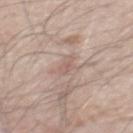workup: catalogued during a skin exam; not biopsied
imaging modality: ~15 mm tile from a whole-body skin photo
automated metrics: an average lesion color of about L≈59 a*≈18 b*≈24 (CIELAB) and about 8 CIELAB-L* units darker than the surrounding skin
lighting: white-light
size: about 2.5 mm
anatomic site: the mid back
patient: male, approximately 50 years of age